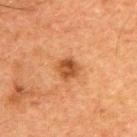Impression:
Part of a total-body skin-imaging series; this lesion was reviewed on a skin check and was not flagged for biopsy.
Context:
Cropped from a total-body skin-imaging series; the visible field is about 15 mm. On the upper back. The lesion's longest dimension is about 3 mm. Captured under cross-polarized illumination. A male subject in their 50s.